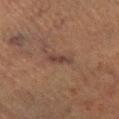biopsy status — imaged on a skin check; not biopsied
subject — male, aged approximately 75
body site — the leg
lighting — cross-polarized
imaging modality — ~15 mm crop, total-body skin-cancer survey
lesion diameter — ≈3 mm